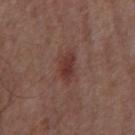The lesion was tiled from a total-body skin photograph and was not biopsied.
A male subject, roughly 55 years of age.
The lesion is located on the chest.
Cropped from a whole-body photographic skin survey; the tile spans about 15 mm.